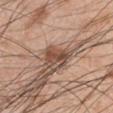| key | value |
|---|---|
| workup | catalogued during a skin exam; not biopsied |